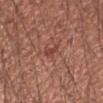biopsy status: imaged on a skin check; not biopsied
patient: male, aged 58 to 62
image: total-body-photography crop, ~15 mm field of view
TBP lesion metrics: a footprint of about 3 mm² and an eccentricity of roughly 0.9; a lesion color around L≈44 a*≈25 b*≈26 in CIELAB, about 7 CIELAB-L* units darker than the surrounding skin, and a lesion-to-skin contrast of about 6 (normalized; higher = more distinct)
size: ~3 mm (longest diameter)
illumination: white-light illumination
body site: the right forearm